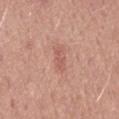biopsy status: catalogued during a skin exam; not biopsied | site: the mid back | illumination: white-light | image: ~15 mm crop, total-body skin-cancer survey | subject: male, aged 48–52.